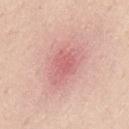biopsy status: catalogued during a skin exam; not biopsied
image: total-body-photography crop, ~15 mm field of view
patient: male, aged around 35
body site: the back